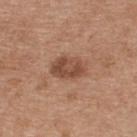Impression: Part of a total-body skin-imaging series; this lesion was reviewed on a skin check and was not flagged for biopsy. Image and clinical context: A female patient in their 40s. The tile uses white-light illumination. A lesion tile, about 15 mm wide, cut from a 3D total-body photograph. The lesion is on the upper back. An algorithmic analysis of the crop reported a within-lesion color-variation index near 4/10 and radial color variation of about 1.5. The software also gave a nevus-likeness score of about 40/100 and lesion-presence confidence of about 100/100.